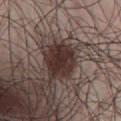Clinical impression: This lesion was catalogued during total-body skin photography and was not selected for biopsy. Acquisition and patient details: The lesion is on the abdomen. The lesion-visualizer software estimated a footprint of about 14 mm², a shape eccentricity near 0.4, and two-axis asymmetry of about 0.2. It also reported a border-irregularity index near 2/10 and internal color variation of about 4 on a 0–10 scale. The software also gave a nevus-likeness score of about 90/100 and lesion-presence confidence of about 100/100. Imaged with white-light lighting. The subject is a male in their mid-40s. A close-up tile cropped from a whole-body skin photograph, about 15 mm across. The recorded lesion diameter is about 4 mm.